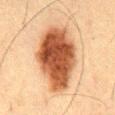Captured during whole-body skin photography for melanoma surveillance; the lesion was not biopsied. Cropped from a total-body skin-imaging series; the visible field is about 15 mm. From the abdomen. The subject is a male approximately 60 years of age.